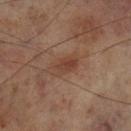The lesion was tiled from a total-body skin photograph and was not biopsied.
This is a cross-polarized tile.
The lesion is on the leg.
A 15 mm close-up extracted from a 3D total-body photography capture.
The patient is a male about 70 years old.
Measured at roughly 3.5 mm in maximum diameter.